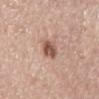biopsy_status: not biopsied; imaged during a skin examination
site: mid back
lesion_size:
  long_diameter_mm_approx: 3.5
lighting: white-light
image:
  source: total-body photography crop
  field_of_view_mm: 15
patient:
  sex: male
  age_approx: 80